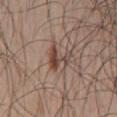biopsy status = no biopsy performed (imaged during a skin exam)
TBP lesion metrics = a footprint of about 6.5 mm² and two-axis asymmetry of about 0.65; a border-irregularity index near 8/10, a within-lesion color-variation index near 4/10, and peripheral color asymmetry of about 1; a classifier nevus-likeness of about 75/100
anatomic site = the mid back
patient = male, aged 48–52
acquisition = 15 mm crop, total-body photography
lesion diameter = ~3.5 mm (longest diameter)